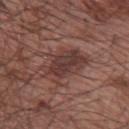Assessment: The lesion was photographed on a routine skin check and not biopsied; there is no pathology result. Image and clinical context: The recorded lesion diameter is about 5 mm. Cropped from a whole-body photographic skin survey; the tile spans about 15 mm. The total-body-photography lesion software estimated an area of roughly 14 mm², an eccentricity of roughly 0.65, and a symmetry-axis asymmetry near 0.25. It also reported border irregularity of about 4.5 on a 0–10 scale and a within-lesion color-variation index near 3.5/10. This is a white-light tile. The subject is a male in their mid-60s. From the arm.